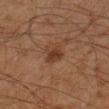Findings:
* follow-up — total-body-photography surveillance lesion; no biopsy
* diameter — about 2.5 mm
* patient — male, in their mid- to late 40s
* illumination — cross-polarized
* acquisition — 15 mm crop, total-body photography
* body site — the right forearm